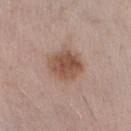Assessment:
Imaged during a routine full-body skin examination; the lesion was not biopsied and no histopathology is available.
Clinical summary:
On the leg. Longest diameter approximately 4.5 mm. Cropped from a total-body skin-imaging series; the visible field is about 15 mm. Imaged with white-light lighting. A female patient aged 28–32. Automated image analysis of the tile measured a lesion area of about 13 mm², an eccentricity of roughly 0.6, and two-axis asymmetry of about 0.15. The analysis additionally found an average lesion color of about L≈52 a*≈19 b*≈28 (CIELAB) and a lesion-to-skin contrast of about 8.5 (normalized; higher = more distinct).